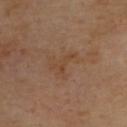Impression: This lesion was catalogued during total-body skin photography and was not selected for biopsy. Image and clinical context: Captured under cross-polarized illumination. Approximately 3 mm at its widest. A lesion tile, about 15 mm wide, cut from a 3D total-body photograph. From the upper back. Automated image analysis of the tile measured an area of roughly 3 mm², an outline eccentricity of about 0.9 (0 = round, 1 = elongated), and a shape-asymmetry score of about 0.6 (0 = symmetric). It also reported border irregularity of about 8 on a 0–10 scale, a within-lesion color-variation index near 0/10, and radial color variation of about 0. The analysis additionally found an automated nevus-likeness rating near 0 out of 100 and a lesion-detection confidence of about 100/100. A male subject, aged 53 to 57.